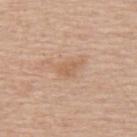| field | value |
|---|---|
| follow-up | total-body-photography surveillance lesion; no biopsy |
| lighting | white-light illumination |
| subject | male, aged approximately 60 |
| site | the upper back |
| imaging modality | ~15 mm crop, total-body skin-cancer survey |
| lesion size | ≈3 mm |
| automated lesion analysis | an average lesion color of about L≈60 a*≈19 b*≈33 (CIELAB) and a lesion–skin lightness drop of about 7; border irregularity of about 3 on a 0–10 scale and internal color variation of about 1 on a 0–10 scale |